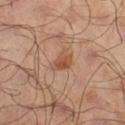The lesion was photographed on a routine skin check and not biopsied; there is no pathology result.
A 15 mm close-up extracted from a 3D total-body photography capture.
The lesion is located on the right lower leg.
A male subject, aged 63–67.
Captured under cross-polarized illumination.